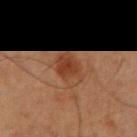The lesion was photographed on a routine skin check and not biopsied; there is no pathology result. A 15 mm crop from a total-body photograph taken for skin-cancer surveillance. The total-body-photography lesion software estimated an average lesion color of about L≈31 a*≈18 b*≈26 (CIELAB), roughly 7 lightness units darker than nearby skin, and a lesion-to-skin contrast of about 7 (normalized; higher = more distinct). And it measured a border-irregularity index near 1.5/10, a within-lesion color-variation index near 4/10, and a peripheral color-asymmetry measure near 1.5. This is a cross-polarized tile. On the right upper arm. The patient is a male aged approximately 60. The recorded lesion diameter is about 4 mm.